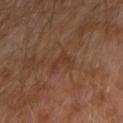biopsy_status: not biopsied; imaged during a skin examination
patient:
  sex: male
  age_approx: 30
automated_metrics:
  shape_asymmetry: 0.55
  cielab_L: 35
  cielab_a: 19
  cielab_b: 27
  vs_skin_darker_L: 5.0
  vs_skin_contrast_norm: 5.0
  peripheral_color_asymmetry: 0.0
  nevus_likeness_0_100: 0
  lesion_detection_confidence_0_100: 100
site: left arm
image:
  source: total-body photography crop
  field_of_view_mm: 15